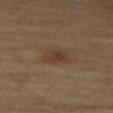Impression: Captured during whole-body skin photography for melanoma surveillance; the lesion was not biopsied. Acquisition and patient details: Longest diameter approximately 3.5 mm. Located on the right thigh. The total-body-photography lesion software estimated a nevus-likeness score of about 50/100 and a lesion-detection confidence of about 100/100. A 15 mm close-up extracted from a 3D total-body photography capture. This is a cross-polarized tile. A female patient aged 58–62.